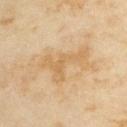<tbp_lesion>
  <automated_metrics>
    <area_mm2_approx>10.0</area_mm2_approx>
    <eccentricity>0.8</eccentricity>
    <shape_asymmetry>0.7</shape_asymmetry>
    <cielab_L>63</cielab_L>
    <cielab_a>15</cielab_a>
    <cielab_b>39</cielab_b>
    <vs_skin_darker_L>7.0</vs_skin_darker_L>
    <vs_skin_contrast_norm>5.5</vs_skin_contrast_norm>
    <border_irregularity_0_10>9.0</border_irregularity_0_10>
    <color_variation_0_10>1.5</color_variation_0_10>
    <peripheral_color_asymmetry>0.5</peripheral_color_asymmetry>
    <nevus_likeness_0_100>0</nevus_likeness_0_100>
    <lesion_detection_confidence_0_100>100</lesion_detection_confidence_0_100>
  </automated_metrics>
  <lighting>cross-polarized</lighting>
  <site>back</site>
  <patient>
    <sex>female</sex>
    <age_approx>35</age_approx>
  </patient>
  <lesion_size>
    <long_diameter_mm_approx>6.0</long_diameter_mm_approx>
  </lesion_size>
  <image>
    <source>total-body photography crop</source>
    <field_of_view_mm>15</field_of_view_mm>
  </image>
</tbp_lesion>